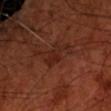follow-up = imaged on a skin check; not biopsied
tile lighting = cross-polarized
diameter = ≈3.5 mm
acquisition = ~15 mm crop, total-body skin-cancer survey
patient = male, aged 68 to 72
location = the front of the torso